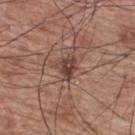Q: Was this lesion biopsied?
A: no biopsy performed (imaged during a skin exam)
Q: Automated lesion metrics?
A: a footprint of about 5 mm² and a shape eccentricity near 0.65; lesion-presence confidence of about 100/100
Q: Lesion location?
A: the upper back
Q: What is the imaging modality?
A: ~15 mm crop, total-body skin-cancer survey
Q: What lighting was used for the tile?
A: white-light
Q: Who is the patient?
A: male, in their 70s